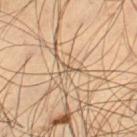Recorded during total-body skin imaging; not selected for excision or biopsy. Automated image analysis of the tile measured an outline eccentricity of about 0.75 (0 = round, 1 = elongated) and two-axis asymmetry of about 0.45. The lesion's longest dimension is about 1.5 mm. The lesion is on the left thigh. Cropped from a total-body skin-imaging series; the visible field is about 15 mm. A male subject aged 43–47. The tile uses cross-polarized illumination.